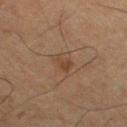Impression:
The lesion was photographed on a routine skin check and not biopsied; there is no pathology result.
Clinical summary:
The subject is a male in their mid-60s. Located on the right thigh. A lesion tile, about 15 mm wide, cut from a 3D total-body photograph. Imaged with cross-polarized lighting. Longest diameter approximately 3 mm. The lesion-visualizer software estimated an average lesion color of about L≈34 a*≈15 b*≈25 (CIELAB), a lesion–skin lightness drop of about 5, and a normalized lesion–skin contrast near 6. The software also gave a detector confidence of about 100 out of 100 that the crop contains a lesion.